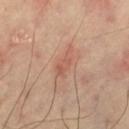This lesion was catalogued during total-body skin photography and was not selected for biopsy. A lesion tile, about 15 mm wide, cut from a 3D total-body photograph. From the left thigh. This is a cross-polarized tile.